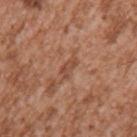The subject is a male approximately 45 years of age. Captured under white-light illumination. The lesion is located on the left upper arm. A lesion tile, about 15 mm wide, cut from a 3D total-body photograph. About 3 mm across.